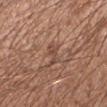This lesion was catalogued during total-body skin photography and was not selected for biopsy. Automated tile analysis of the lesion measured a lesion area of about 3.5 mm² and a shape-asymmetry score of about 0.4 (0 = symmetric). The analysis additionally found roughly 7 lightness units darker than nearby skin and a normalized lesion–skin contrast near 5.5. It also reported a border-irregularity index near 4.5/10, internal color variation of about 0.5 on a 0–10 scale, and peripheral color asymmetry of about 0. The patient is a male in their 30s. Cropped from a whole-body photographic skin survey; the tile spans about 15 mm. About 3 mm across. Located on the left forearm.